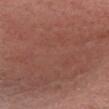The lesion was tiled from a total-body skin photograph and was not biopsied. Captured under white-light illumination. This image is a 15 mm lesion crop taken from a total-body photograph. A female patient, aged approximately 55. Automated tile analysis of the lesion measured a lesion color around L≈48 a*≈22 b*≈25 in CIELAB, roughly 8 lightness units darker than nearby skin, and a normalized lesion–skin contrast near 6. The analysis additionally found a border-irregularity index near 5/10, a within-lesion color-variation index near 5/10, and radial color variation of about 1.5. The lesion's longest dimension is about 19 mm. Located on the chest.